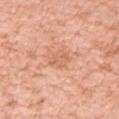Clinical impression: Recorded during total-body skin imaging; not selected for excision or biopsy. Image and clinical context: The patient is a female aged around 40. A roughly 15 mm field-of-view crop from a total-body skin photograph. The lesion is located on the right forearm. The tile uses white-light illumination. Measured at roughly 3 mm in maximum diameter.